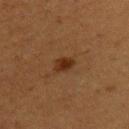notes: no biopsy performed (imaged during a skin exam) | subject: female, about 40 years old | tile lighting: cross-polarized | image-analysis metrics: a shape eccentricity near 0.8 and a shape-asymmetry score of about 0.25 (0 = symmetric); a mean CIELAB color near L≈25 a*≈18 b*≈28, about 8 CIELAB-L* units darker than the surrounding skin, and a normalized border contrast of about 9.5; a color-variation rating of about 2/10 and a peripheral color-asymmetry measure near 0.5; a classifier nevus-likeness of about 90/100 and a detector confidence of about 100 out of 100 that the crop contains a lesion | body site: the left upper arm | lesion size: ~2.5 mm (longest diameter) | acquisition: total-body-photography crop, ~15 mm field of view.